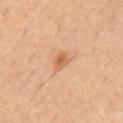follow-up = no biopsy performed (imaged during a skin exam); size = about 2.5 mm; subject = male, about 70 years old; location = the abdomen; imaging modality = ~15 mm crop, total-body skin-cancer survey.